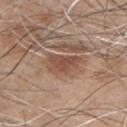• notes — catalogued during a skin exam; not biopsied
• image source — 15 mm crop, total-body photography
• location — the chest
• patient — male, roughly 45 years of age